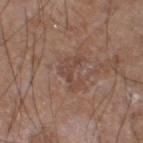This lesion was catalogued during total-body skin photography and was not selected for biopsy.
A male subject in their 60s.
A roughly 15 mm field-of-view crop from a total-body skin photograph.
Located on the left lower leg.